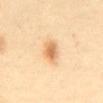Notes:
* workup · imaged on a skin check; not biopsied
* subject · female, aged around 40
* image · ~15 mm crop, total-body skin-cancer survey
* image-analysis metrics · a footprint of about 6 mm² and an outline eccentricity of about 0.9 (0 = round, 1 = elongated); a mean CIELAB color near L≈73 a*≈21 b*≈42, roughly 13 lightness units darker than nearby skin, and a normalized lesion–skin contrast near 7.5; a border-irregularity rating of about 2.5/10, internal color variation of about 4.5 on a 0–10 scale, and radial color variation of about 1.5
* site · the back
* tile lighting · cross-polarized
* diameter · ≈4 mm